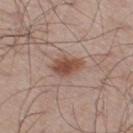Recorded during total-body skin imaging; not selected for excision or biopsy. The recorded lesion diameter is about 4 mm. A male subject in their 60s. Located on the right thigh. A 15 mm crop from a total-body photograph taken for skin-cancer surveillance. Imaged with white-light lighting.